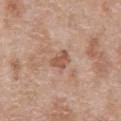The lesion was tiled from a total-body skin photograph and was not biopsied.
Imaged with white-light lighting.
A roughly 15 mm field-of-view crop from a total-body skin photograph.
Measured at roughly 3 mm in maximum diameter.
On the front of the torso.
The subject is a male in their 50s.
An algorithmic analysis of the crop reported an average lesion color of about L≈55 a*≈20 b*≈30 (CIELAB), roughly 10 lightness units darker than nearby skin, and a lesion-to-skin contrast of about 7 (normalized; higher = more distinct). The analysis additionally found a border-irregularity index near 3/10, a color-variation rating of about 1.5/10, and a peripheral color-asymmetry measure near 0.5.